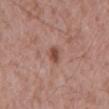Q: Was this lesion biopsied?
A: no biopsy performed (imaged during a skin exam)
Q: What kind of image is this?
A: ~15 mm crop, total-body skin-cancer survey
Q: What lighting was used for the tile?
A: white-light illumination
Q: What are the patient's age and sex?
A: male, aged around 60
Q: Lesion size?
A: about 2.5 mm
Q: Lesion location?
A: the mid back